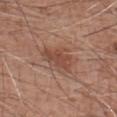<case>
<site>left forearm</site>
<automated_metrics>
  <eccentricity>0.7</eccentricity>
  <shape_asymmetry>0.25</shape_asymmetry>
  <cielab_L>47</cielab_L>
  <cielab_a>22</cielab_a>
  <cielab_b>28</cielab_b>
  <vs_skin_darker_L>8.0</vs_skin_darker_L>
  <vs_skin_contrast_norm>6.5</vs_skin_contrast_norm>
  <nevus_likeness_0_100>20</nevus_likeness_0_100>
  <lesion_detection_confidence_0_100>100</lesion_detection_confidence_0_100>
</automated_metrics>
<image>
  <source>total-body photography crop</source>
  <field_of_view_mm>15</field_of_view_mm>
</image>
<patient>
  <sex>male</sex>
  <age_approx>60</age_approx>
</patient>
<lesion_size>
  <long_diameter_mm_approx>4.0</long_diameter_mm_approx>
</lesion_size>
<lighting>white-light</lighting>
</case>